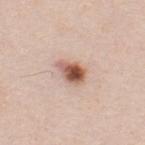Clinical impression:
The lesion was tiled from a total-body skin photograph and was not biopsied.
Clinical summary:
The lesion is on the upper back. A male subject, about 35 years old. Cropped from a total-body skin-imaging series; the visible field is about 15 mm.